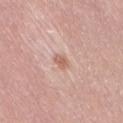Clinical impression:
Recorded during total-body skin imaging; not selected for excision or biopsy.
Image and clinical context:
The recorded lesion diameter is about 2 mm. A 15 mm crop from a total-body photograph taken for skin-cancer surveillance. The patient is a female approximately 40 years of age. The tile uses white-light illumination. Automated image analysis of the tile measured a lesion area of about 2.5 mm², an eccentricity of roughly 0.7, and a shape-asymmetry score of about 0.25 (0 = symmetric). The software also gave a mean CIELAB color near L≈62 a*≈22 b*≈28, about 9 CIELAB-L* units darker than the surrounding skin, and a normalized lesion–skin contrast near 6.5. And it measured an automated nevus-likeness rating near 10 out of 100. The lesion is located on the lower back.